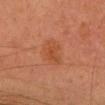Recorded during total-body skin imaging; not selected for excision or biopsy.
Cropped from a total-body skin-imaging series; the visible field is about 15 mm.
A female patient, aged approximately 20.
Automated image analysis of the tile measured an average lesion color of about L≈43 a*≈24 b*≈33 (CIELAB) and a normalized lesion–skin contrast near 5.5. The analysis additionally found border irregularity of about 2.5 on a 0–10 scale, a color-variation rating of about 2.5/10, and radial color variation of about 1. It also reported a nevus-likeness score of about 20/100 and lesion-presence confidence of about 100/100.
The tile uses cross-polarized illumination.
The lesion's longest dimension is about 4 mm.
The lesion is located on the head or neck.